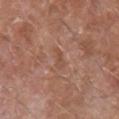biopsy status = no biopsy performed (imaged during a skin exam)
illumination = white-light illumination
acquisition = ~15 mm crop, total-body skin-cancer survey
subject = male, in their 80s
diameter = ~2.5 mm (longest diameter)
location = the left upper arm